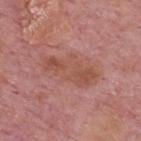Notes:
• workup: total-body-photography surveillance lesion; no biopsy
• subject: male, aged 73 to 77
• lesion size: about 6.5 mm
• automated metrics: a lesion color around L≈51 a*≈24 b*≈28 in CIELAB, about 7 CIELAB-L* units darker than the surrounding skin, and a lesion-to-skin contrast of about 6 (normalized; higher = more distinct); a border-irregularity index near 4/10, a color-variation rating of about 3/10, and peripheral color asymmetry of about 1
• image: 15 mm crop, total-body photography
• anatomic site: the upper back